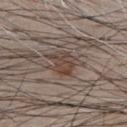Impression:
No biopsy was performed on this lesion — it was imaged during a full skin examination and was not determined to be concerning.
Context:
A 15 mm close-up tile from a total-body photography series done for melanoma screening. The patient is a male aged 78 to 82. The recorded lesion diameter is about 4.5 mm. The lesion is on the front of the torso.